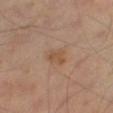workup = imaged on a skin check; not biopsied.